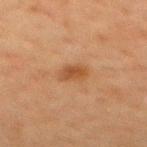Q: Is there a histopathology result?
A: total-body-photography surveillance lesion; no biopsy
Q: How was this image acquired?
A: 15 mm crop, total-body photography
Q: What is the anatomic site?
A: the mid back
Q: Who is the patient?
A: male, roughly 60 years of age
Q: Lesion size?
A: ≈3 mm
Q: What did automated image analysis measure?
A: an area of roughly 5 mm² and an eccentricity of roughly 0.75
Q: Illumination type?
A: cross-polarized